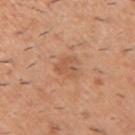Imaged during a routine full-body skin examination; the lesion was not biopsied and no histopathology is available. Approximately 3 mm at its widest. The patient is a male aged approximately 40. This image is a 15 mm lesion crop taken from a total-body photograph. Located on the right upper arm. This is a white-light tile.